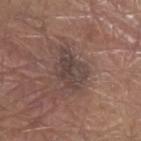follow-up: total-body-photography surveillance lesion; no biopsy | illumination: white-light | automated lesion analysis: a border-irregularity rating of about 4/10, a color-variation rating of about 4/10, and peripheral color asymmetry of about 1.5; a lesion-detection confidence of about 80/100 | site: the left forearm | size: ~5 mm (longest diameter) | image source: total-body-photography crop, ~15 mm field of view | subject: male, in their 80s.